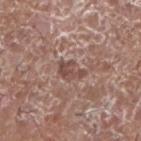| key | value |
|---|---|
| biopsy status | catalogued during a skin exam; not biopsied |
| illumination | white-light illumination |
| body site | the right upper arm |
| TBP lesion metrics | a lesion color around L≈47 a*≈19 b*≈23 in CIELAB and a lesion–skin lightness drop of about 9; border irregularity of about 5.5 on a 0–10 scale and a peripheral color-asymmetry measure near 0.5; a classifier nevus-likeness of about 0/100 and lesion-presence confidence of about 90/100 |
| imaging modality | 15 mm crop, total-body photography |
| subject | male, in their mid- to late 70s |